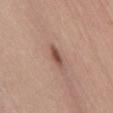follow-up=imaged on a skin check; not biopsied
imaging modality=~15 mm tile from a whole-body skin photo
tile lighting=white-light
patient=male, in their 60s
body site=the abdomen
automated metrics=a mean CIELAB color near L≈51 a*≈21 b*≈26, about 12 CIELAB-L* units darker than the surrounding skin, and a normalized lesion–skin contrast near 8.5